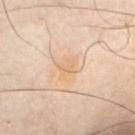biopsy_status: not biopsied; imaged during a skin examination